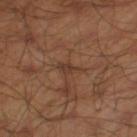Impression:
No biopsy was performed on this lesion — it was imaged during a full skin examination and was not determined to be concerning.
Background:
This is a cross-polarized tile. The lesion is located on the leg. Approximately 2.5 mm at its widest. A male patient, in their mid- to late 40s. The total-body-photography lesion software estimated a lesion color around L≈36 a*≈18 b*≈27 in CIELAB, roughly 6 lightness units darker than nearby skin, and a lesion-to-skin contrast of about 6 (normalized; higher = more distinct). The software also gave a nevus-likeness score of about 0/100 and lesion-presence confidence of about 50/100. A 15 mm close-up tile from a total-body photography series done for melanoma screening.